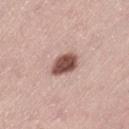Automated image analysis of the tile measured a lesion–skin lightness drop of about 19 and a lesion-to-skin contrast of about 12 (normalized; higher = more distinct). It also reported a classifier nevus-likeness of about 90/100. From the left thigh. The lesion's longest dimension is about 3.5 mm. A female subject, aged approximately 40. A close-up tile cropped from a whole-body skin photograph, about 15 mm across. This is a white-light tile.